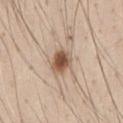Impression:
Captured during whole-body skin photography for melanoma surveillance; the lesion was not biopsied.
Background:
Measured at roughly 3.5 mm in maximum diameter. The lesion-visualizer software estimated a lesion color around L≈55 a*≈17 b*≈30 in CIELAB and a lesion–skin lightness drop of about 15. It also reported border irregularity of about 1.5 on a 0–10 scale, internal color variation of about 5 on a 0–10 scale, and a peripheral color-asymmetry measure near 1.5. Captured under white-light illumination. The patient is a male aged 43 to 47. The lesion is located on the front of the torso. A lesion tile, about 15 mm wide, cut from a 3D total-body photograph.